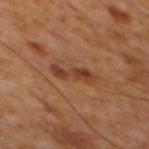Background: Measured at roughly 4.5 mm in maximum diameter. The lesion is on the mid back. A 15 mm close-up tile from a total-body photography series done for melanoma screening. A male subject aged around 65.